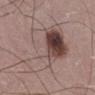| field | value |
|---|---|
| follow-up | imaged on a skin check; not biopsied |
| illumination | white-light illumination |
| patient | male, aged around 50 |
| automated lesion analysis | an average lesion color of about L≈49 a*≈16 b*≈20 (CIELAB), a lesion–skin lightness drop of about 9, and a lesion-to-skin contrast of about 7 (normalized; higher = more distinct); a detector confidence of about 100 out of 100 that the crop contains a lesion |
| imaging modality | ~15 mm crop, total-body skin-cancer survey |
| location | the right lower leg |
| size | ~9 mm (longest diameter) |